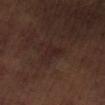Assessment:
This lesion was catalogued during total-body skin photography and was not selected for biopsy.
Context:
A male patient, in their 50s. The recorded lesion diameter is about 3.5 mm. A 15 mm close-up tile from a total-body photography series done for melanoma screening. On the right lower leg. Captured under cross-polarized illumination.